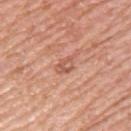A region of skin cropped from a whole-body photographic capture, roughly 15 mm wide. Automated image analysis of the tile measured a border-irregularity index near 2.5/10, a color-variation rating of about 3.5/10, and a peripheral color-asymmetry measure near 1. It also reported a nevus-likeness score of about 0/100 and lesion-presence confidence of about 100/100. About 2.5 mm across. On the upper back. A female patient, roughly 40 years of age. Captured under white-light illumination.